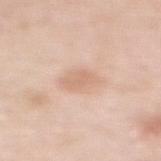The lesion was photographed on a routine skin check and not biopsied; there is no pathology result.
From the upper back.
The tile uses white-light illumination.
A female patient in their 50s.
Automated image analysis of the tile measured a lesion color around L≈69 a*≈19 b*≈30 in CIELAB and roughly 8 lightness units darker than nearby skin.
A 15 mm crop from a total-body photograph taken for skin-cancer surveillance.
About 3.5 mm across.